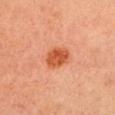Image and clinical context:
A female subject aged 18–22. Imaged with cross-polarized lighting. The lesion is located on the head or neck. Approximately 3.5 mm at its widest. A region of skin cropped from a whole-body photographic capture, roughly 15 mm wide.